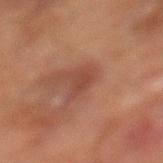Imaged during a routine full-body skin examination; the lesion was not biopsied and no histopathology is available. This image is a 15 mm lesion crop taken from a total-body photograph. Captured under cross-polarized illumination. A male subject, in their 70s. The lesion-visualizer software estimated a lesion area of about 3.5 mm², an eccentricity of roughly 0.7, and a shape-asymmetry score of about 0.35 (0 = symmetric). It also reported a border-irregularity rating of about 3/10 and a within-lesion color-variation index near 1.5/10. It also reported a nevus-likeness score of about 0/100. The lesion is on the left lower leg. The recorded lesion diameter is about 2.5 mm.